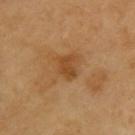| field | value |
|---|---|
| workup | total-body-photography surveillance lesion; no biopsy |
| imaging modality | ~15 mm crop, total-body skin-cancer survey |
| automated metrics | a footprint of about 4.5 mm², an eccentricity of roughly 0.7, and two-axis asymmetry of about 0.3; a border-irregularity index near 3/10, internal color variation of about 1.5 on a 0–10 scale, and radial color variation of about 0.5; a nevus-likeness score of about 15/100 |
| lesion diameter | ≈2.5 mm |
| lighting | cross-polarized |
| location | the upper back |
| subject | male, aged 58 to 62 |